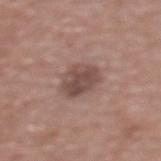A 15 mm crop from a total-body photograph taken for skin-cancer surveillance. The patient is a female aged 68 to 72. Automated tile analysis of the lesion measured an area of roughly 10 mm² and two-axis asymmetry of about 0.15. And it measured about 11 CIELAB-L* units darker than the surrounding skin and a lesion-to-skin contrast of about 8 (normalized; higher = more distinct). The software also gave border irregularity of about 1.5 on a 0–10 scale and a within-lesion color-variation index near 3.5/10. And it measured lesion-presence confidence of about 100/100. On the upper back. Approximately 4 mm at its widest.